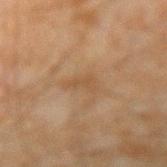Assessment:
Recorded during total-body skin imaging; not selected for excision or biopsy.
Clinical summary:
From the right forearm. A male subject aged approximately 45. Imaged with cross-polarized lighting. A close-up tile cropped from a whole-body skin photograph, about 15 mm across. Longest diameter approximately 2.5 mm.